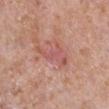biopsy_status: not biopsied; imaged during a skin examination
patient:
  sex: female
  age_approx: 40
site: right lower leg
image:
  source: total-body photography crop
  field_of_view_mm: 15